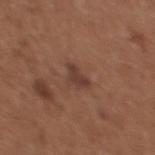Assessment: Part of a total-body skin-imaging series; this lesion was reviewed on a skin check and was not flagged for biopsy. Background: Longest diameter approximately 2.5 mm. The total-body-photography lesion software estimated a footprint of about 3.5 mm², an eccentricity of roughly 0.75, and a symmetry-axis asymmetry near 0.4. The software also gave a border-irregularity index near 4/10 and a peripheral color-asymmetry measure near 0.5. A lesion tile, about 15 mm wide, cut from a 3D total-body photograph. The tile uses white-light illumination. A female patient, roughly 35 years of age. From the front of the torso.